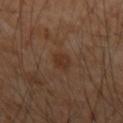biopsy_status: not biopsied; imaged during a skin examination